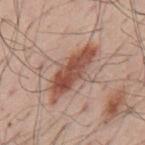Case summary:
• follow-up · total-body-photography surveillance lesion; no biopsy
• image · 15 mm crop, total-body photography
• diameter · ≈7.5 mm
• patient · male, in their mid-50s
• image-analysis metrics · an average lesion color of about L≈51 a*≈22 b*≈28 (CIELAB), about 13 CIELAB-L* units darker than the surrounding skin, and a normalized border contrast of about 9.5; border irregularity of about 4 on a 0–10 scale and internal color variation of about 4.5 on a 0–10 scale
• site · the back
• tile lighting · white-light